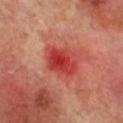Q: Was this lesion biopsied?
A: catalogued during a skin exam; not biopsied
Q: How was the tile lit?
A: cross-polarized illumination
Q: What is the imaging modality?
A: 15 mm crop, total-body photography
Q: What did automated image analysis measure?
A: a shape eccentricity near 0.65 and two-axis asymmetry of about 0.2; an average lesion color of about L≈44 a*≈42 b*≈32 (CIELAB) and roughly 11 lightness units darker than nearby skin; a border-irregularity index near 2/10 and peripheral color asymmetry of about 1.5; a classifier nevus-likeness of about 0/100
Q: Where on the body is the lesion?
A: the right lower leg
Q: Who is the patient?
A: male, aged approximately 70